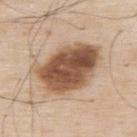The lesion was tiled from a total-body skin photograph and was not biopsied. The subject is a male aged 78 to 82. A 15 mm crop from a total-body photograph taken for skin-cancer surveillance. This is a white-light tile. On the back.